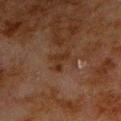Recorded during total-body skin imaging; not selected for excision or biopsy.
A male patient aged around 80.
Located on the front of the torso.
A 15 mm close-up extracted from a 3D total-body photography capture.